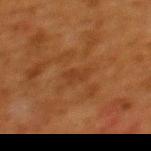Imaged during a routine full-body skin examination; the lesion was not biopsied and no histopathology is available.
On the upper back.
An algorithmic analysis of the crop reported a lesion area of about 3 mm², an outline eccentricity of about 0.8 (0 = round, 1 = elongated), and a symmetry-axis asymmetry near 0.35. It also reported an average lesion color of about L≈31 a*≈20 b*≈31 (CIELAB), roughly 5 lightness units darker than nearby skin, and a normalized lesion–skin contrast near 5.
The recorded lesion diameter is about 2.5 mm.
Captured under cross-polarized illumination.
A female subject, roughly 50 years of age.
A lesion tile, about 15 mm wide, cut from a 3D total-body photograph.